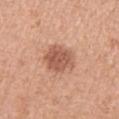Imaged during a routine full-body skin examination; the lesion was not biopsied and no histopathology is available. Located on the right upper arm. Automated image analysis of the tile measured a footprint of about 11 mm² and a shape-asymmetry score of about 0.2 (0 = symmetric). And it measured a lesion color around L≈56 a*≈24 b*≈31 in CIELAB and a normalized border contrast of about 8. And it measured lesion-presence confidence of about 100/100. A close-up tile cropped from a whole-body skin photograph, about 15 mm across. Approximately 4 mm at its widest. A female patient, aged approximately 60.